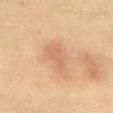Impression: Part of a total-body skin-imaging series; this lesion was reviewed on a skin check and was not flagged for biopsy. Image and clinical context: The recorded lesion diameter is about 4 mm. Automated image analysis of the tile measured about 6 CIELAB-L* units darker than the surrounding skin and a lesion-to-skin contrast of about 4.5 (normalized; higher = more distinct). The patient is a female aged around 55. The lesion is located on the abdomen. A roughly 15 mm field-of-view crop from a total-body skin photograph. Captured under cross-polarized illumination.